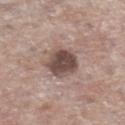The lesion was tiled from a total-body skin photograph and was not biopsied.
Cropped from a whole-body photographic skin survey; the tile spans about 15 mm.
An algorithmic analysis of the crop reported an outline eccentricity of about 0.5 (0 = round, 1 = elongated) and a shape-asymmetry score of about 0.2 (0 = symmetric). It also reported an average lesion color of about L≈47 a*≈16 b*≈21 (CIELAB) and a lesion–skin lightness drop of about 15. The analysis additionally found border irregularity of about 2 on a 0–10 scale and internal color variation of about 4 on a 0–10 scale. And it measured an automated nevus-likeness rating near 45 out of 100.
This is a white-light tile.
On the right lower leg.
A female subject, aged 83 or over.